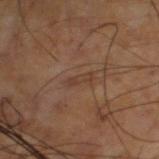Case summary:
- follow-up: catalogued during a skin exam; not biopsied